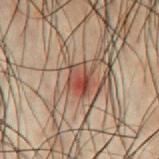Clinical impression: Part of a total-body skin-imaging series; this lesion was reviewed on a skin check and was not flagged for biopsy. Acquisition and patient details: About 3 mm across. The subject is a male roughly 50 years of age. The lesion-visualizer software estimated an average lesion color of about L≈35 a*≈20 b*≈23 (CIELAB), about 8 CIELAB-L* units darker than the surrounding skin, and a normalized lesion–skin contrast near 7.5. It also reported border irregularity of about 2 on a 0–10 scale, internal color variation of about 8.5 on a 0–10 scale, and radial color variation of about 3.5. A 15 mm crop from a total-body photograph taken for skin-cancer surveillance. Imaged with cross-polarized lighting. The lesion is located on the front of the torso.